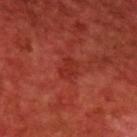Q: How large is the lesion?
A: about 2.5 mm
Q: What kind of image is this?
A: ~15 mm crop, total-body skin-cancer survey
Q: How was the tile lit?
A: cross-polarized illumination
Q: What are the patient's age and sex?
A: male, aged around 60
Q: Lesion location?
A: the upper back